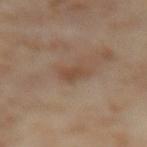A lesion tile, about 15 mm wide, cut from a 3D total-body photograph. The subject is a female roughly 55 years of age. On the left thigh.